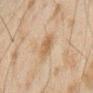<case>
  <biopsy_status>not biopsied; imaged during a skin examination</biopsy_status>
  <lesion_size>
    <long_diameter_mm_approx>3.0</long_diameter_mm_approx>
  </lesion_size>
  <image>
    <source>total-body photography crop</source>
    <field_of_view_mm>15</field_of_view_mm>
  </image>
  <lighting>cross-polarized</lighting>
  <patient>
    <sex>male</sex>
    <age_approx>45</age_approx>
  </patient>
  <site>arm</site>
</case>